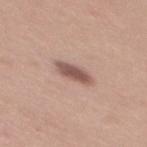Notes:
– image: ~15 mm tile from a whole-body skin photo
– site: the mid back
– patient: female, about 25 years old